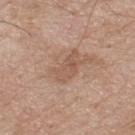Case summary:
* biopsy status: total-body-photography surveillance lesion; no biopsy
* body site: the upper back
* lesion size: about 3.5 mm
* tile lighting: white-light illumination
* subject: male, roughly 75 years of age
* image: total-body-photography crop, ~15 mm field of view
* automated metrics: a lesion area of about 7 mm² and an outline eccentricity of about 0.8 (0 = round, 1 = elongated)